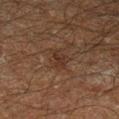Assessment: Part of a total-body skin-imaging series; this lesion was reviewed on a skin check and was not flagged for biopsy. Image and clinical context: Captured under cross-polarized illumination. Cropped from a total-body skin-imaging series; the visible field is about 15 mm. The lesion's longest dimension is about 2.5 mm. A male patient, approximately 60 years of age. Located on the left lower leg.